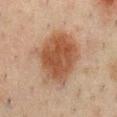{
  "biopsy_status": "not biopsied; imaged during a skin examination",
  "site": "chest",
  "patient": {
    "sex": "male",
    "age_approx": 50
  },
  "image": {
    "source": "total-body photography crop",
    "field_of_view_mm": 15
  }
}